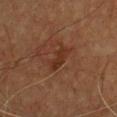Assessment:
The lesion was photographed on a routine skin check and not biopsied; there is no pathology result.
Background:
On the front of the torso. A male subject, in their mid-60s. Imaged with cross-polarized lighting. The recorded lesion diameter is about 3.5 mm. A 15 mm close-up tile from a total-body photography series done for melanoma screening. The total-body-photography lesion software estimated an average lesion color of about L≈26 a*≈18 b*≈25 (CIELAB) and roughly 6 lightness units darker than nearby skin. The software also gave a classifier nevus-likeness of about 0/100 and lesion-presence confidence of about 100/100.